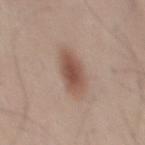This lesion was catalogued during total-body skin photography and was not selected for biopsy.
A 15 mm close-up tile from a total-body photography series done for melanoma screening.
The subject is a male in their mid- to late 30s.
Longest diameter approximately 4.5 mm.
The lesion is located on the mid back.
Imaged with white-light lighting.
Automated tile analysis of the lesion measured a lesion area of about 10 mm² and a shape-asymmetry score of about 0.15 (0 = symmetric). The software also gave border irregularity of about 1.5 on a 0–10 scale and a peripheral color-asymmetry measure near 1.